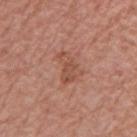workup=imaged on a skin check; not biopsied.